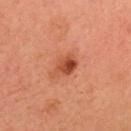biopsy status: no biopsy performed (imaged during a skin exam)
image source: total-body-photography crop, ~15 mm field of view
anatomic site: the head or neck
patient: male, aged approximately 35